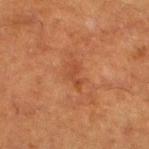Impression:
The lesion was photographed on a routine skin check and not biopsied; there is no pathology result.
Clinical summary:
The lesion-visualizer software estimated a border-irregularity rating of about 6/10, a within-lesion color-variation index near 0/10, and peripheral color asymmetry of about 0. And it measured a classifier nevus-likeness of about 0/100 and a lesion-detection confidence of about 100/100. The lesion is located on the right lower leg. A male subject, approximately 65 years of age. Imaged with cross-polarized lighting. A 15 mm crop from a total-body photograph taken for skin-cancer surveillance.